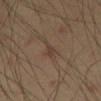Q: Was a biopsy performed?
A: no biopsy performed (imaged during a skin exam)
Q: What is the imaging modality?
A: 15 mm crop, total-body photography
Q: What is the anatomic site?
A: the left thigh
Q: Patient demographics?
A: male, roughly 40 years of age